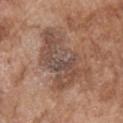<tbp_lesion>
<biopsy_status>not biopsied; imaged during a skin examination</biopsy_status>
<lighting>white-light</lighting>
<image>
  <source>total-body photography crop</source>
  <field_of_view_mm>15</field_of_view_mm>
</image>
<patient>
  <sex>male</sex>
  <age_approx>75</age_approx>
</patient>
<site>arm</site>
<lesion_size>
  <long_diameter_mm_approx>8.5</long_diameter_mm_approx>
</lesion_size>
</tbp_lesion>